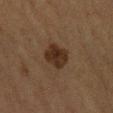Impression:
The lesion was tiled from a total-body skin photograph and was not biopsied.
Image and clinical context:
Approximately 3.5 mm at its widest. Automated image analysis of the tile measured a lesion area of about 8.5 mm², a shape eccentricity near 0.65, and a symmetry-axis asymmetry near 0.15. And it measured a border-irregularity index near 1.5/10, a within-lesion color-variation index near 3/10, and a peripheral color-asymmetry measure near 1. The software also gave a nevus-likeness score of about 80/100 and a detector confidence of about 100 out of 100 that the crop contains a lesion. Cropped from a total-body skin-imaging series; the visible field is about 15 mm. This is a cross-polarized tile. The patient is a male aged 58 to 62. The lesion is located on the left forearm.